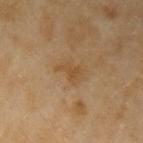- follow-up: catalogued during a skin exam; not biopsied
- subject: female, approximately 60 years of age
- tile lighting: cross-polarized
- acquisition: ~15 mm tile from a whole-body skin photo
- body site: the left upper arm
- automated lesion analysis: an area of roughly 3.5 mm², an outline eccentricity of about 0.8 (0 = round, 1 = elongated), and two-axis asymmetry of about 0.55; an average lesion color of about L≈44 a*≈16 b*≈34 (CIELAB), a lesion–skin lightness drop of about 6, and a normalized border contrast of about 6; a border-irregularity rating of about 5.5/10, a color-variation rating of about 0.5/10, and peripheral color asymmetry of about 0.5; an automated nevus-likeness rating near 0 out of 100 and a detector confidence of about 100 out of 100 that the crop contains a lesion
- lesion size: ~3 mm (longest diameter)